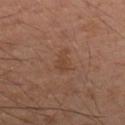<case>
<biopsy_status>not biopsied; imaged during a skin examination</biopsy_status>
<image>
  <source>total-body photography crop</source>
  <field_of_view_mm>15</field_of_view_mm>
</image>
<patient>
  <sex>male</sex>
  <age_approx>45</age_approx>
</patient>
<site>right forearm</site>
</case>